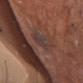biopsy_status: not biopsied; imaged during a skin examination
patient:
  sex: male
  age_approx: 80
site: head or neck
image:
  source: total-body photography crop
  field_of_view_mm: 15
lighting: white-light
lesion_size:
  long_diameter_mm_approx: 4.0